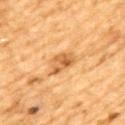biopsy_status: not biopsied; imaged during a skin examination
image:
  source: total-body photography crop
  field_of_view_mm: 15
site: mid back
lighting: cross-polarized
lesion_size:
  long_diameter_mm_approx: 4.0
patient:
  sex: male
  age_approx: 85+